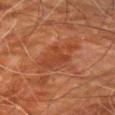<lesion>
<biopsy_status>not biopsied; imaged during a skin examination</biopsy_status>
<image>
  <source>total-body photography crop</source>
  <field_of_view_mm>15</field_of_view_mm>
</image>
<patient>
  <sex>male</sex>
  <age_approx>80</age_approx>
</patient>
<lesion_size>
  <long_diameter_mm_approx>5.0</long_diameter_mm_approx>
</lesion_size>
<site>right upper arm</site>
</lesion>